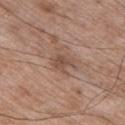Q: Is there a histopathology result?
A: total-body-photography surveillance lesion; no biopsy